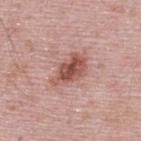Recorded during total-body skin imaging; not selected for excision or biopsy. The total-body-photography lesion software estimated border irregularity of about 3 on a 0–10 scale, a color-variation rating of about 5/10, and radial color variation of about 1.5. On the upper back. Measured at roughly 4.5 mm in maximum diameter. The patient is a male in their 50s. A close-up tile cropped from a whole-body skin photograph, about 15 mm across. Imaged with white-light lighting.